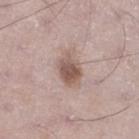Background:
A close-up tile cropped from a whole-body skin photograph, about 15 mm across. From the left lower leg. Imaged with white-light lighting. A male subject roughly 70 years of age. Longest diameter approximately 4 mm.